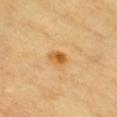{"biopsy_status": "not biopsied; imaged during a skin examination", "site": "front of the torso", "patient": {"sex": "male", "age_approx": 60}, "lighting": "cross-polarized", "image": {"source": "total-body photography crop", "field_of_view_mm": 15}, "automated_metrics": {"color_variation_0_10": 5.0, "peripheral_color_asymmetry": 1.5}, "lesion_size": {"long_diameter_mm_approx": 2.5}}